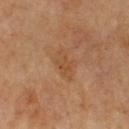| feature | finding |
|---|---|
| patient | male, roughly 65 years of age |
| image source | total-body-photography crop, ~15 mm field of view |
| body site | the front of the torso |